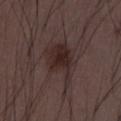Recorded during total-body skin imaging; not selected for excision or biopsy.
A male patient, approximately 30 years of age.
Approximately 6 mm at its widest.
From the abdomen.
Cropped from a whole-body photographic skin survey; the tile spans about 15 mm.